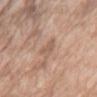{"biopsy_status": "not biopsied; imaged during a skin examination", "patient": {"sex": "female", "age_approx": 75}, "site": "arm", "image": {"source": "total-body photography crop", "field_of_view_mm": 15}}